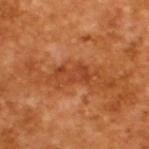Q: Was a biopsy performed?
A: imaged on a skin check; not biopsied
Q: What is the imaging modality?
A: total-body-photography crop, ~15 mm field of view
Q: Patient demographics?
A: male, about 65 years old
Q: How was the tile lit?
A: cross-polarized illumination
Q: How large is the lesion?
A: ~4.5 mm (longest diameter)